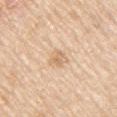Q: Was this lesion biopsied?
A: total-body-photography surveillance lesion; no biopsy
Q: What is the lesion's diameter?
A: about 2.5 mm
Q: What kind of image is this?
A: total-body-photography crop, ~15 mm field of view
Q: What is the anatomic site?
A: the right upper arm
Q: What did automated image analysis measure?
A: a lesion area of about 4.5 mm², an eccentricity of roughly 0.6, and two-axis asymmetry of about 0.25; a border-irregularity index near 2/10, internal color variation of about 3.5 on a 0–10 scale, and a peripheral color-asymmetry measure near 1.5; an automated nevus-likeness rating near 0 out of 100 and lesion-presence confidence of about 100/100
Q: What are the patient's age and sex?
A: male, in their 60s